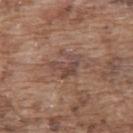Clinical impression: This lesion was catalogued during total-body skin photography and was not selected for biopsy. Acquisition and patient details: A roughly 15 mm field-of-view crop from a total-body skin photograph. Longest diameter approximately 4 mm. A male subject aged approximately 75. Located on the upper back. Imaged with white-light lighting.